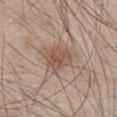Imaged during a routine full-body skin examination; the lesion was not biopsied and no histopathology is available. The tile uses white-light illumination. A 15 mm close-up tile from a total-body photography series done for melanoma screening. The total-body-photography lesion software estimated a footprint of about 7.5 mm² and an outline eccentricity of about 0.45 (0 = round, 1 = elongated). And it measured a border-irregularity rating of about 2/10, a within-lesion color-variation index near 3.5/10, and peripheral color asymmetry of about 1. The software also gave an automated nevus-likeness rating near 80 out of 100 and a detector confidence of about 100 out of 100 that the crop contains a lesion. A male subject aged 63 to 67. From the chest.